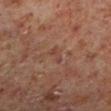Q: Was this lesion biopsied?
A: total-body-photography surveillance lesion; no biopsy
Q: Where on the body is the lesion?
A: the left lower leg
Q: What are the patient's age and sex?
A: male, aged around 60
Q: How was this image acquired?
A: total-body-photography crop, ~15 mm field of view
Q: Lesion size?
A: ≈2.5 mm